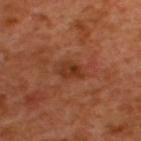Q: Was a biopsy performed?
A: total-body-photography surveillance lesion; no biopsy
Q: What is the anatomic site?
A: the upper back
Q: Lesion size?
A: ~3 mm (longest diameter)
Q: Automated lesion metrics?
A: an eccentricity of roughly 0.75; a mean CIELAB color near L≈34 a*≈26 b*≈33, a lesion–skin lightness drop of about 8, and a lesion-to-skin contrast of about 7.5 (normalized; higher = more distinct); a within-lesion color-variation index near 4.5/10 and radial color variation of about 1.5
Q: What are the patient's age and sex?
A: male, aged 48–52
Q: What kind of image is this?
A: 15 mm crop, total-body photography